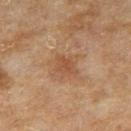Recorded during total-body skin imaging; not selected for excision or biopsy.
A 15 mm crop from a total-body photograph taken for skin-cancer surveillance.
The total-body-photography lesion software estimated a border-irregularity rating of about 2/10 and internal color variation of about 1.5 on a 0–10 scale.
The lesion is located on the right thigh.
The patient is a female aged 58 to 62.
Captured under cross-polarized illumination.